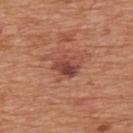Q: Was this lesion biopsied?
A: total-body-photography surveillance lesion; no biopsy
Q: What are the patient's age and sex?
A: male, aged 63 to 67
Q: What did automated image analysis measure?
A: a footprint of about 5.5 mm², a shape eccentricity near 0.65, and two-axis asymmetry of about 0.2; a classifier nevus-likeness of about 65/100 and a detector confidence of about 100 out of 100 that the crop contains a lesion
Q: What is the imaging modality?
A: ~15 mm tile from a whole-body skin photo
Q: How was the tile lit?
A: white-light illumination
Q: What is the anatomic site?
A: the upper back